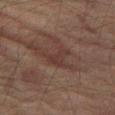Background: A close-up tile cropped from a whole-body skin photograph, about 15 mm across. Located on the right thigh. The tile uses cross-polarized illumination. A male patient aged around 75. Automated image analysis of the tile measured an automated nevus-likeness rating near 0 out of 100 and a lesion-detection confidence of about 60/100. Measured at roughly 3.5 mm in maximum diameter.